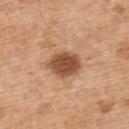Assessment:
Part of a total-body skin-imaging series; this lesion was reviewed on a skin check and was not flagged for biopsy.
Image and clinical context:
The subject is a male aged 53 to 57. A lesion tile, about 15 mm wide, cut from a 3D total-body photograph. On the upper back.